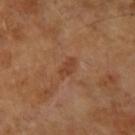No biopsy was performed on this lesion — it was imaged during a full skin examination and was not determined to be concerning. A lesion tile, about 15 mm wide, cut from a 3D total-body photograph. The lesion is located on the right forearm. A female subject aged 68–72. Longest diameter approximately 2.5 mm. An algorithmic analysis of the crop reported a border-irregularity rating of about 2.5/10, internal color variation of about 2.5 on a 0–10 scale, and radial color variation of about 1. It also reported a nevus-likeness score of about 0/100. Captured under cross-polarized illumination.